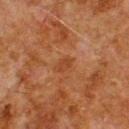Impression: Recorded during total-body skin imaging; not selected for excision or biopsy. Image and clinical context: Imaged with cross-polarized lighting. A region of skin cropped from a whole-body photographic capture, roughly 15 mm wide. A male subject, aged approximately 80. The lesion is on the upper back.